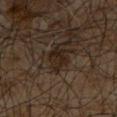Clinical impression: Part of a total-body skin-imaging series; this lesion was reviewed on a skin check and was not flagged for biopsy. Clinical summary: Located on the upper back. Approximately 3.5 mm at its widest. Cropped from a whole-body photographic skin survey; the tile spans about 15 mm. A male subject, aged 63 to 67. An algorithmic analysis of the crop reported a lesion–skin lightness drop of about 7. The analysis additionally found a border-irregularity rating of about 4/10, internal color variation of about 2.5 on a 0–10 scale, and a peripheral color-asymmetry measure near 1. This is a cross-polarized tile.